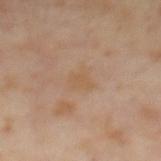illumination: cross-polarized illumination
TBP lesion metrics: a lesion color around L≈54 a*≈16 b*≈31 in CIELAB, a lesion–skin lightness drop of about 4, and a normalized border contrast of about 5; border irregularity of about 3.5 on a 0–10 scale, internal color variation of about 1.5 on a 0–10 scale, and peripheral color asymmetry of about 0.5; a detector confidence of about 100 out of 100 that the crop contains a lesion
imaging modality: 15 mm crop, total-body photography
lesion size: about 3 mm
patient: male, aged approximately 65